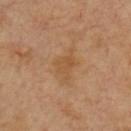– biopsy status — imaged on a skin check; not biopsied
– subject — male, in their mid-60s
– illumination — cross-polarized
– acquisition — total-body-photography crop, ~15 mm field of view